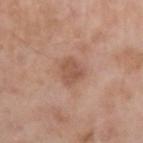biopsy_status: not biopsied; imaged during a skin examination
automated_metrics:
  nevus_likeness_0_100: 10
  lesion_detection_confidence_0_100: 100
site: left upper arm
patient:
  sex: male
  age_approx: 55
image:
  source: total-body photography crop
  field_of_view_mm: 15
lesion_size:
  long_diameter_mm_approx: 3.0
lighting: white-light